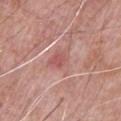<lesion>
  <image>
    <source>total-body photography crop</source>
    <field_of_view_mm>15</field_of_view_mm>
  </image>
  <automated_metrics>
    <area_mm2_approx>7.5</area_mm2_approx>
    <eccentricity>0.35</eccentricity>
    <shape_asymmetry>0.35</shape_asymmetry>
  </automated_metrics>
  <patient>
    <sex>male</sex>
    <age_approx>70</age_approx>
  </patient>
  <site>chest</site>
  <lighting>white-light</lighting>
</lesion>